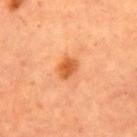Q: Is there a histopathology result?
A: total-body-photography surveillance lesion; no biopsy
Q: What is the imaging modality?
A: ~15 mm crop, total-body skin-cancer survey
Q: Lesion size?
A: about 2.5 mm
Q: Illumination type?
A: cross-polarized illumination
Q: Lesion location?
A: the right upper arm
Q: Who is the patient?
A: female, in their 60s